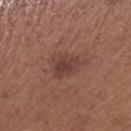Q: Patient demographics?
A: female, about 55 years old
Q: How was this image acquired?
A: 15 mm crop, total-body photography
Q: Where on the body is the lesion?
A: the leg
Q: How large is the lesion?
A: ~3.5 mm (longest diameter)